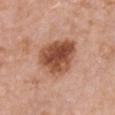Impression: No biopsy was performed on this lesion — it was imaged during a full skin examination and was not determined to be concerning. Context: Automated image analysis of the tile measured a nevus-likeness score of about 80/100. A female subject, aged 48–52. On the chest. Longest diameter approximately 5.5 mm. A region of skin cropped from a whole-body photographic capture, roughly 15 mm wide.